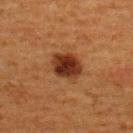  biopsy_status: not biopsied; imaged during a skin examination
  site: upper back
  image:
    source: total-body photography crop
    field_of_view_mm: 15
  automated_metrics:
    shape_asymmetry: 0.15
    vs_skin_darker_L: 13.0
    border_irregularity_0_10: 1.5
    color_variation_0_10: 4.0
    peripheral_color_asymmetry: 1.0
    nevus_likeness_0_100: 100
    lesion_detection_confidence_0_100: 100
  patient:
    sex: female
    age_approx: 40